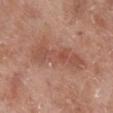patient: male, roughly 75 years of age | lesion diameter: about 7.5 mm | image: ~15 mm tile from a whole-body skin photo | anatomic site: the right lower leg | TBP lesion metrics: a footprint of about 17 mm², an eccentricity of roughly 0.95, and a shape-asymmetry score of about 0.35 (0 = symmetric).